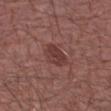• follow-up · total-body-photography surveillance lesion; no biopsy
• site · the right forearm
• size · about 3.5 mm
• image · total-body-photography crop, ~15 mm field of view
• illumination · white-light illumination
• subject · male, about 65 years old